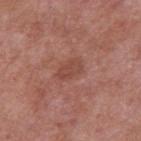image source: ~15 mm tile from a whole-body skin photo
anatomic site: the right upper arm
illumination: white-light illumination
diameter: ≈3 mm
image-analysis metrics: an area of roughly 4 mm², an outline eccentricity of about 0.8 (0 = round, 1 = elongated), and a shape-asymmetry score of about 0.15 (0 = symmetric); a lesion–skin lightness drop of about 7 and a normalized lesion–skin contrast near 5.5; a nevus-likeness score of about 0/100 and a detector confidence of about 100 out of 100 that the crop contains a lesion
subject: male, roughly 55 years of age